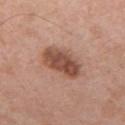Recorded during total-body skin imaging; not selected for excision or biopsy. About 5.5 mm across. Imaged with white-light lighting. A 15 mm crop from a total-body photograph taken for skin-cancer surveillance. The total-body-photography lesion software estimated an average lesion color of about L≈50 a*≈22 b*≈28 (CIELAB), about 13 CIELAB-L* units darker than the surrounding skin, and a normalized border contrast of about 9.5. And it measured an automated nevus-likeness rating near 70 out of 100 and lesion-presence confidence of about 100/100. From the chest. A male subject, aged 53 to 57.